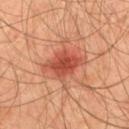<record>
<biopsy_status>not biopsied; imaged during a skin examination</biopsy_status>
<lighting>cross-polarized</lighting>
<automated_metrics>
  <area_mm2_approx>15.0</area_mm2_approx>
  <eccentricity>0.75</eccentricity>
  <shape_asymmetry>0.35</shape_asymmetry>
  <cielab_L>50</cielab_L>
  <cielab_a>30</cielab_a>
  <cielab_b>32</cielab_b>
  <vs_skin_contrast_norm>8.0</vs_skin_contrast_norm>
  <nevus_likeness_0_100>85</nevus_likeness_0_100>
  <lesion_detection_confidence_0_100>100</lesion_detection_confidence_0_100>
</automated_metrics>
<site>mid back</site>
<patient>
  <sex>male</sex>
  <age_approx>45</age_approx>
</patient>
<lesion_size>
  <long_diameter_mm_approx>5.5</long_diameter_mm_approx>
</lesion_size>
<image>
  <source>total-body photography crop</source>
  <field_of_view_mm>15</field_of_view_mm>
</image>
</record>